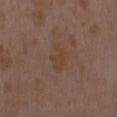Q: What is the anatomic site?
A: the front of the torso
Q: Automated lesion metrics?
A: an outline eccentricity of about 0.85 (0 = round, 1 = elongated); a mean CIELAB color near L≈40 a*≈16 b*≈26, a lesion–skin lightness drop of about 4, and a normalized border contrast of about 5; a border-irregularity rating of about 4/10 and peripheral color asymmetry of about 0.5; a lesion-detection confidence of about 100/100
Q: Lesion size?
A: about 3.5 mm
Q: Illumination type?
A: white-light
Q: Who is the patient?
A: female, aged 28–32
Q: What kind of image is this?
A: ~15 mm tile from a whole-body skin photo